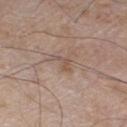<tbp_lesion>
  <lighting>white-light</lighting>
  <patient>
    <sex>male</sex>
    <age_approx>55</age_approx>
  </patient>
  <image>
    <source>total-body photography crop</source>
    <field_of_view_mm>15</field_of_view_mm>
  </image>
  <lesion_size>
    <long_diameter_mm_approx>2.5</long_diameter_mm_approx>
  </lesion_size>
  <automated_metrics>
    <area_mm2_approx>3.0</area_mm2_approx>
    <eccentricity>0.8</eccentricity>
    <shape_asymmetry>0.5</shape_asymmetry>
    <cielab_L>53</cielab_L>
    <cielab_a>16</cielab_a>
    <cielab_b>25</cielab_b>
    <vs_skin_darker_L>7.0</vs_skin_darker_L>
  </automated_metrics>
  <site>front of the torso</site>
</tbp_lesion>